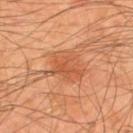Impression: Imaged during a routine full-body skin examination; the lesion was not biopsied and no histopathology is available. Image and clinical context: This is a cross-polarized tile. From the back. A male subject approximately 45 years of age. Automated tile analysis of the lesion measured a footprint of about 9.5 mm², a shape eccentricity near 0.55, and two-axis asymmetry of about 0.4. And it measured a mean CIELAB color near L≈52 a*≈27 b*≈37, roughly 8 lightness units darker than nearby skin, and a normalized lesion–skin contrast near 6. And it measured border irregularity of about 4.5 on a 0–10 scale, internal color variation of about 3 on a 0–10 scale, and peripheral color asymmetry of about 1. A roughly 15 mm field-of-view crop from a total-body skin photograph.